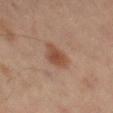Assessment: This lesion was catalogued during total-body skin photography and was not selected for biopsy. Acquisition and patient details: Measured at roughly 3.5 mm in maximum diameter. The subject is a male approximately 60 years of age. Cropped from a whole-body photographic skin survey; the tile spans about 15 mm. From the leg. This is a cross-polarized tile.